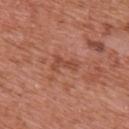Impression:
The lesion was tiled from a total-body skin photograph and was not biopsied.
Background:
A male patient, about 70 years old. A 15 mm close-up tile from a total-body photography series done for melanoma screening. The lesion's longest dimension is about 3 mm. From the upper back. Imaged with white-light lighting.